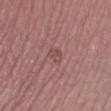| field | value |
|---|---|
| biopsy status | catalogued during a skin exam; not biopsied |
| image-analysis metrics | an average lesion color of about L≈48 a*≈23 b*≈23 (CIELAB), roughly 6 lightness units darker than nearby skin, and a normalized border contrast of about 5.5; a border-irregularity index near 2/10 and radial color variation of about 0.5; a classifier nevus-likeness of about 0/100 |
| body site | the right lower leg |
| tile lighting | white-light illumination |
| patient | female, approximately 50 years of age |
| image | total-body-photography crop, ~15 mm field of view |
| size | ≈2.5 mm |